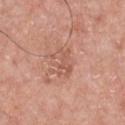No biopsy was performed on this lesion — it was imaged during a full skin examination and was not determined to be concerning. The tile uses white-light illumination. The lesion is located on the chest. An algorithmic analysis of the crop reported a lesion color around L≈57 a*≈24 b*≈29 in CIELAB, a lesion–skin lightness drop of about 7, and a lesion-to-skin contrast of about 4.5 (normalized; higher = more distinct). And it measured border irregularity of about 4 on a 0–10 scale, a color-variation rating of about 4.5/10, and a peripheral color-asymmetry measure near 1.5. It also reported a classifier nevus-likeness of about 0/100. A male patient aged around 60. A 15 mm crop from a total-body photograph taken for skin-cancer surveillance.